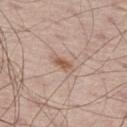Case summary:
• follow-up: catalogued during a skin exam; not biopsied
• subject: male, in their mid-60s
• tile lighting: white-light illumination
• anatomic site: the left thigh
• acquisition: total-body-photography crop, ~15 mm field of view